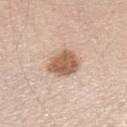No biopsy was performed on this lesion — it was imaged during a full skin examination and was not determined to be concerning.